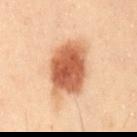Assessment:
Captured during whole-body skin photography for melanoma surveillance; the lesion was not biopsied.
Acquisition and patient details:
The patient is a male aged approximately 55. Cropped from a whole-body photographic skin survey; the tile spans about 15 mm. Imaged with cross-polarized lighting. The lesion is on the mid back. Automated image analysis of the tile measured a footprint of about 22 mm², a shape eccentricity near 0.7, and a shape-asymmetry score of about 0.2 (0 = symmetric). The analysis additionally found a mean CIELAB color near L≈48 a*≈22 b*≈31, a lesion–skin lightness drop of about 15, and a normalized border contrast of about 11. The software also gave an automated nevus-likeness rating near 100 out of 100 and a lesion-detection confidence of about 100/100. About 6 mm across.